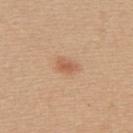Clinical summary: Automated tile analysis of the lesion measured a shape eccentricity near 0.8 and a symmetry-axis asymmetry near 0.25. The software also gave an average lesion color of about L≈58 a*≈22 b*≈35 (CIELAB), roughly 9 lightness units darker than nearby skin, and a lesion-to-skin contrast of about 6.5 (normalized; higher = more distinct). It also reported a detector confidence of about 100 out of 100 that the crop contains a lesion. Imaged with white-light lighting. A female subject aged around 40. The recorded lesion diameter is about 2.5 mm. A 15 mm crop from a total-body photograph taken for skin-cancer surveillance. The lesion is located on the upper back.